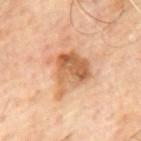Impression: The lesion was tiled from a total-body skin photograph and was not biopsied. Context: About 5 mm across. The tile uses cross-polarized illumination. A male subject aged around 65. Located on the mid back. A 15 mm close-up extracted from a 3D total-body photography capture.